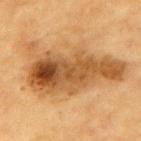workup — catalogued during a skin exam; not biopsied
patient — male, roughly 85 years of age or older
location — the upper back
imaging modality — total-body-photography crop, ~15 mm field of view
lighting — cross-polarized
image-analysis metrics — an area of roughly 45 mm², an outline eccentricity of about 0.9 (0 = round, 1 = elongated), and a symmetry-axis asymmetry near 0.35; a border-irregularity rating of about 5.5/10, a color-variation rating of about 9/10, and radial color variation of about 3